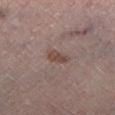{
  "biopsy_status": "not biopsied; imaged during a skin examination",
  "image": {
    "source": "total-body photography crop",
    "field_of_view_mm": 15
  },
  "site": "leg",
  "patient": {
    "sex": "male",
    "age_approx": 70
  },
  "lighting": "cross-polarized"
}